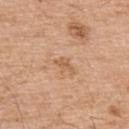A male patient in their mid-50s. The tile uses white-light illumination. Measured at roughly 3 mm in maximum diameter. A 15 mm crop from a total-body photograph taken for skin-cancer surveillance. Automated image analysis of the tile measured an area of roughly 3 mm², an outline eccentricity of about 0.85 (0 = round, 1 = elongated), and a shape-asymmetry score of about 0.45 (0 = symmetric). It also reported an average lesion color of about L≈60 a*≈21 b*≈35 (CIELAB), a lesion–skin lightness drop of about 8, and a normalized lesion–skin contrast near 5.5. It also reported a border-irregularity index near 4.5/10, a within-lesion color-variation index near 1/10, and radial color variation of about 0.5. And it measured an automated nevus-likeness rating near 0 out of 100 and lesion-presence confidence of about 100/100. On the upper back.